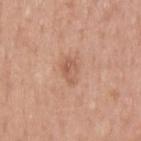Imaged during a routine full-body skin examination; the lesion was not biopsied and no histopathology is available.
From the upper back.
Automated image analysis of the tile measured a footprint of about 5 mm², a shape eccentricity near 0.8, and a symmetry-axis asymmetry near 0.2. The software also gave roughly 8 lightness units darker than nearby skin and a normalized border contrast of about 5.5. It also reported a nevus-likeness score of about 0/100 and lesion-presence confidence of about 100/100.
A 15 mm close-up extracted from a 3D total-body photography capture.
Imaged with white-light lighting.
A male subject, aged approximately 40.
Measured at roughly 3 mm in maximum diameter.